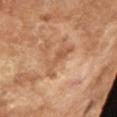| feature | finding |
|---|---|
| image | ~15 mm crop, total-body skin-cancer survey |
| size | ≈4 mm |
| lighting | cross-polarized illumination |
| subject | male, aged 63–67 |